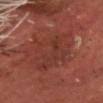Q: Was a biopsy performed?
A: imaged on a skin check; not biopsied
Q: Patient demographics?
A: male, approximately 70 years of age
Q: Lesion location?
A: the head or neck
Q: What is the imaging modality?
A: ~15 mm crop, total-body skin-cancer survey